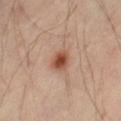<record>
<biopsy_status>not biopsied; imaged during a skin examination</biopsy_status>
<patient>
  <sex>male</sex>
  <age_approx>55</age_approx>
</patient>
<image>
  <source>total-body photography crop</source>
  <field_of_view_mm>15</field_of_view_mm>
</image>
<site>right thigh</site>
</record>